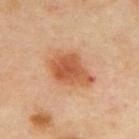Q: Is there a histopathology result?
A: imaged on a skin check; not biopsied
Q: How large is the lesion?
A: ≈5.5 mm
Q: Patient demographics?
A: female, approximately 40 years of age
Q: What is the imaging modality?
A: total-body-photography crop, ~15 mm field of view
Q: What did automated image analysis measure?
A: an area of roughly 15 mm², a shape eccentricity near 0.75, and a shape-asymmetry score of about 0.25 (0 = symmetric); a nevus-likeness score of about 100/100 and a detector confidence of about 100 out of 100 that the crop contains a lesion
Q: Lesion location?
A: the upper back